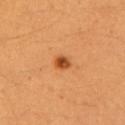Case summary:
- workup: no biopsy performed (imaged during a skin exam)
- automated metrics: a mean CIELAB color near L≈51 a*≈30 b*≈45 and about 14 CIELAB-L* units darker than the surrounding skin; radial color variation of about 1.5
- body site: the left upper arm
- diameter: about 2 mm
- patient: female, in their 40s
- tile lighting: cross-polarized illumination
- imaging modality: ~15 mm tile from a whole-body skin photo